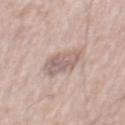Impression: Recorded during total-body skin imaging; not selected for excision or biopsy. Background: A male patient, aged approximately 75. Cropped from a whole-body photographic skin survey; the tile spans about 15 mm. The lesion is on the mid back. The lesion-visualizer software estimated a footprint of about 10 mm² and a shape-asymmetry score of about 0.25 (0 = symmetric). The analysis additionally found a lesion color around L≈61 a*≈16 b*≈21 in CIELAB, roughly 10 lightness units darker than nearby skin, and a normalized border contrast of about 6.5. The software also gave a nevus-likeness score of about 0/100.